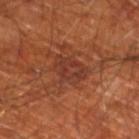Imaged during a routine full-body skin examination; the lesion was not biopsied and no histopathology is available. Automated tile analysis of the lesion measured an average lesion color of about L≈35 a*≈25 b*≈29 (CIELAB) and about 7 CIELAB-L* units darker than the surrounding skin. It also reported a border-irregularity rating of about 3/10, a within-lesion color-variation index near 3.5/10, and radial color variation of about 1. The lesion is on the left lower leg. A 15 mm crop from a total-body photograph taken for skin-cancer surveillance. A male subject, about 70 years old. Measured at roughly 4 mm in maximum diameter.